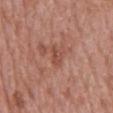No biopsy was performed on this lesion — it was imaged during a full skin examination and was not determined to be concerning.
A lesion tile, about 15 mm wide, cut from a 3D total-body photograph.
The patient is a male in their mid- to late 60s.
Located on the front of the torso.